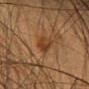Captured during whole-body skin photography for melanoma surveillance; the lesion was not biopsied.
An algorithmic analysis of the crop reported a mean CIELAB color near L≈34 a*≈19 b*≈31, a lesion–skin lightness drop of about 9, and a lesion-to-skin contrast of about 8 (normalized; higher = more distinct). The software also gave a border-irregularity index near 3/10, a color-variation rating of about 3.5/10, and radial color variation of about 1.5.
The subject is a female aged 38–42.
Located on the head or neck.
Captured under cross-polarized illumination.
Longest diameter approximately 3.5 mm.
This image is a 15 mm lesion crop taken from a total-body photograph.